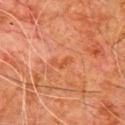image source — total-body-photography crop, ~15 mm field of view; subject — male, roughly 80 years of age; anatomic site — the chest; size — ≈2.5 mm; tile lighting — cross-polarized.